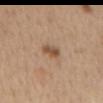Case summary:
– biopsy status: total-body-photography surveillance lesion; no biopsy
– body site: the mid back
– automated metrics: an area of roughly 4 mm²; an average lesion color of about L≈52 a*≈18 b*≈31 (CIELAB), a lesion–skin lightness drop of about 11, and a normalized border contrast of about 8; border irregularity of about 2 on a 0–10 scale and peripheral color asymmetry of about 1; a nevus-likeness score of about 75/100
– lesion diameter: ≈2.5 mm
– subject: male, in their 70s
– acquisition: ~15 mm crop, total-body skin-cancer survey